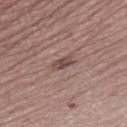illumination — white-light illumination
subject — male, aged approximately 65
image source — total-body-photography crop, ~15 mm field of view
diameter — ~3 mm (longest diameter)
site — the right thigh